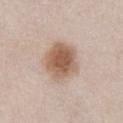No biopsy was performed on this lesion — it was imaged during a full skin examination and was not determined to be concerning.
A 15 mm close-up extracted from a 3D total-body photography capture.
The lesion is on the abdomen.
A female patient aged 73–77.
Imaged with white-light lighting.
An algorithmic analysis of the crop reported a lesion area of about 16 mm², a shape eccentricity near 0.45, and a shape-asymmetry score of about 0.2 (0 = symmetric). The analysis additionally found a border-irregularity rating of about 2/10, internal color variation of about 4.5 on a 0–10 scale, and peripheral color asymmetry of about 1.